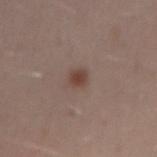Case summary:
– workup: imaged on a skin check; not biopsied
– image: ~15 mm crop, total-body skin-cancer survey
– site: the right upper arm
– patient: male, aged 28 to 32